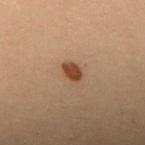lesion diameter: about 2.5 mm
subject: female, aged around 30
anatomic site: the upper back
acquisition: ~15 mm tile from a whole-body skin photo
illumination: cross-polarized